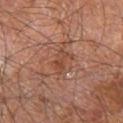Part of a total-body skin-imaging series; this lesion was reviewed on a skin check and was not flagged for biopsy. A lesion tile, about 15 mm wide, cut from a 3D total-body photograph. Imaged with cross-polarized lighting. Automated tile analysis of the lesion measured an area of roughly 4 mm², an outline eccentricity of about 0.8 (0 = round, 1 = elongated), and a shape-asymmetry score of about 0.4 (0 = symmetric). The recorded lesion diameter is about 3 mm. From the right leg. A male subject, aged 58–62.